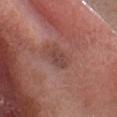Imaged during a routine full-body skin examination; the lesion was not biopsied and no histopathology is available. From the head or neck. A 15 mm close-up extracted from a 3D total-body photography capture. A male subject approximately 35 years of age.